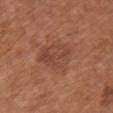– notes · no biopsy performed (imaged during a skin exam)
– body site · the front of the torso
– acquisition · total-body-photography crop, ~15 mm field of view
– patient · female, aged 63 to 67